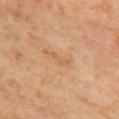follow-up: catalogued during a skin exam; not biopsied
tile lighting: cross-polarized illumination
imaging modality: total-body-photography crop, ~15 mm field of view
subject: female, roughly 45 years of age
anatomic site: the right upper arm
lesion diameter: ~3 mm (longest diameter)